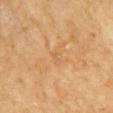The lesion was photographed on a routine skin check and not biopsied; there is no pathology result. Automated tile analysis of the lesion measured two-axis asymmetry of about 0.3. And it measured a lesion color around L≈59 a*≈20 b*≈39 in CIELAB and roughly 5 lightness units darker than nearby skin. The analysis additionally found border irregularity of about 2 on a 0–10 scale, internal color variation of about 1.5 on a 0–10 scale, and a peripheral color-asymmetry measure near 0.5. And it measured a classifier nevus-likeness of about 0/100 and lesion-presence confidence of about 100/100. The subject is a female in their mid-60s. On the right upper arm. A close-up tile cropped from a whole-body skin photograph, about 15 mm across. Longest diameter approximately 1.5 mm. Captured under cross-polarized illumination.